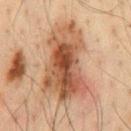workup = catalogued during a skin exam; not biopsied | image = ~15 mm tile from a whole-body skin photo | image-analysis metrics = a border-irregularity rating of about 4/10, a within-lesion color-variation index near 9/10, and a peripheral color-asymmetry measure near 3; an automated nevus-likeness rating near 90 out of 100 and a lesion-detection confidence of about 95/100 | illumination = cross-polarized illumination | size = ~9.5 mm (longest diameter) | anatomic site = the chest | subject = male, about 35 years old.